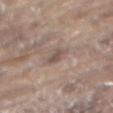Findings:
• lighting — white-light illumination
• anatomic site — the front of the torso
• imaging modality — ~15 mm tile from a whole-body skin photo
• subject — male, aged around 80
• lesion size — ≈2.5 mm
• automated metrics — an automated nevus-likeness rating near 0 out of 100 and a detector confidence of about 95 out of 100 that the crop contains a lesion